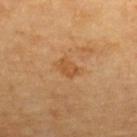Case summary:
* follow-up: imaged on a skin check; not biopsied
* automated lesion analysis: an area of roughly 4 mm², an eccentricity of roughly 0.75, and a shape-asymmetry score of about 0.2 (0 = symmetric)
* subject: roughly 60 years of age
* lighting: cross-polarized illumination
* lesion diameter: about 2.5 mm
* image: 15 mm crop, total-body photography
* location: the back